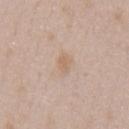Q: Was a biopsy performed?
A: total-body-photography surveillance lesion; no biopsy